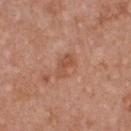– notes · total-body-photography surveillance lesion; no biopsy
– imaging modality · ~15 mm tile from a whole-body skin photo
– lesion size · about 3 mm
– illumination · white-light
– patient · female, aged 38–42
– automated lesion analysis · an area of roughly 4.5 mm², a shape eccentricity near 0.85, and two-axis asymmetry of about 0.3; an automated nevus-likeness rating near 0 out of 100 and a lesion-detection confidence of about 100/100
– site · the chest